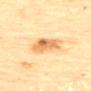No biopsy was performed on this lesion — it was imaged during a full skin examination and was not determined to be concerning. A region of skin cropped from a whole-body photographic capture, roughly 15 mm wide. A female subject, aged 78 to 82. From the back. Measured at roughly 5 mm in maximum diameter. This is a cross-polarized tile.